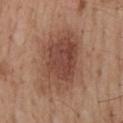{
  "biopsy_status": "not biopsied; imaged during a skin examination",
  "lesion_size": {
    "long_diameter_mm_approx": 8.0
  },
  "image": {
    "source": "total-body photography crop",
    "field_of_view_mm": 15
  },
  "patient": {
    "sex": "male",
    "age_approx": 55
  },
  "site": "mid back",
  "lighting": "white-light",
  "automated_metrics": {
    "eccentricity": 0.7,
    "shape_asymmetry": 0.2
  }
}